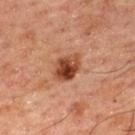The lesion is on the upper back.
A close-up tile cropped from a whole-body skin photograph, about 15 mm across.
A male patient, aged approximately 50.
This is a cross-polarized tile.
Automated image analysis of the tile measured a border-irregularity rating of about 1.5/10 and a peripheral color-asymmetry measure near 2.5.
Approximately 3.5 mm at its widest.